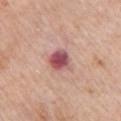biopsy status — no biopsy performed (imaged during a skin exam) | automated metrics — a shape eccentricity near 0.4 and a shape-asymmetry score of about 0.2 (0 = symmetric) | location — the chest | subject — male, approximately 80 years of age | acquisition — total-body-photography crop, ~15 mm field of view | tile lighting — white-light | lesion diameter — about 3 mm.